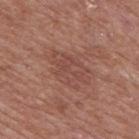Acquisition and patient details: A male patient in their mid-60s. The lesion is on the mid back. A 15 mm close-up extracted from a 3D total-body photography capture. Longest diameter approximately 5.5 mm.